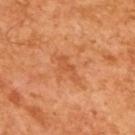  biopsy_status: not biopsied; imaged during a skin examination
  image:
    source: total-body photography crop
    field_of_view_mm: 15
  automated_metrics:
    area_mm2_approx: 4.0
    eccentricity: 0.9
    shape_asymmetry: 0.45
    cielab_L: 54
    cielab_a: 29
    cielab_b: 41
    vs_skin_darker_L: 7.0
    color_variation_0_10: 0.5
    peripheral_color_asymmetry: 0.0
  patient:
    sex: male
    age_approx: 65
  lesion_size:
    long_diameter_mm_approx: 3.5
  lighting: cross-polarized